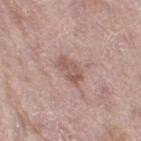biopsy status: no biopsy performed (imaged during a skin exam) | subject: female, aged 68–72 | image: ~15 mm crop, total-body skin-cancer survey | anatomic site: the leg.